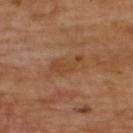Assessment: Part of a total-body skin-imaging series; this lesion was reviewed on a skin check and was not flagged for biopsy. Background: A male subject aged 68 to 72. The tile uses cross-polarized illumination. Measured at roughly 5 mm in maximum diameter. Located on the back. Automated image analysis of the tile measured an average lesion color of about L≈44 a*≈20 b*≈34 (CIELAB) and a lesion–skin lightness drop of about 5. And it measured a border-irregularity index near 4/10, a color-variation rating of about 2.5/10, and peripheral color asymmetry of about 1. A 15 mm close-up tile from a total-body photography series done for melanoma screening.